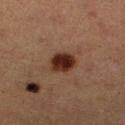<record>
  <lesion_size>
    <long_diameter_mm_approx>3.0</long_diameter_mm_approx>
  </lesion_size>
  <image>
    <source>total-body photography crop</source>
    <field_of_view_mm>15</field_of_view_mm>
  </image>
  <patient>
    <sex>female</sex>
    <age_approx>40</age_approx>
  </patient>
  <site>right lower leg</site>
  <lighting>cross-polarized</lighting>
</record>